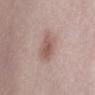A female subject, aged 38 to 42.
From the abdomen.
The tile uses white-light illumination.
The recorded lesion diameter is about 3.5 mm.
A close-up tile cropped from a whole-body skin photograph, about 15 mm across.
The total-body-photography lesion software estimated a footprint of about 7 mm², an outline eccentricity of about 0.7 (0 = round, 1 = elongated), and two-axis asymmetry of about 0.2.